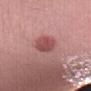Q: Was a biopsy performed?
A: catalogued during a skin exam; not biopsied
Q: What is the anatomic site?
A: the right forearm
Q: Automated lesion metrics?
A: border irregularity of about 1.5 on a 0–10 scale, a color-variation rating of about 3/10, and radial color variation of about 1
Q: What are the patient's age and sex?
A: male, aged approximately 30
Q: What is the imaging modality?
A: ~15 mm crop, total-body skin-cancer survey
Q: Lesion size?
A: ≈3.5 mm